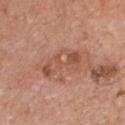lighting=white-light
subject=male, approximately 60 years of age
diameter=about 5.5 mm
anatomic site=the chest
automated metrics=two-axis asymmetry of about 0.4; a mean CIELAB color near L≈53 a*≈24 b*≈30, roughly 8 lightness units darker than nearby skin, and a normalized border contrast of about 6; a classifier nevus-likeness of about 0/100 and a lesion-detection confidence of about 100/100
image source=15 mm crop, total-body photography